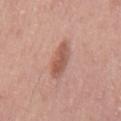follow-up: total-body-photography surveillance lesion; no biopsy | size: ~4 mm (longest diameter) | site: the mid back | automated metrics: a mean CIELAB color near L≈55 a*≈23 b*≈27, about 11 CIELAB-L* units darker than the surrounding skin, and a normalized border contrast of about 7.5; a classifier nevus-likeness of about 90/100 | patient: male, aged around 55 | acquisition: ~15 mm tile from a whole-body skin photo.